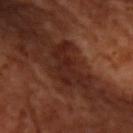Notes:
– image source · 15 mm crop, total-body photography
– size · ≈5.5 mm
– location · the arm
– patient · aged 63 to 67
– tile lighting · cross-polarized illumination